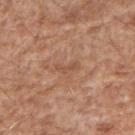No biopsy was performed on this lesion — it was imaged during a full skin examination and was not determined to be concerning.
Located on the arm.
The subject is a male approximately 60 years of age.
A roughly 15 mm field-of-view crop from a total-body skin photograph.
Measured at roughly 2.5 mm in maximum diameter.